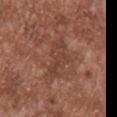Recorded during total-body skin imaging; not selected for excision or biopsy. Captured under white-light illumination. A male patient aged 43 to 47. Cropped from a whole-body photographic skin survey; the tile spans about 15 mm. The lesion-visualizer software estimated an average lesion color of about L≈42 a*≈22 b*≈26 (CIELAB), roughly 7 lightness units darker than nearby skin, and a lesion-to-skin contrast of about 5.5 (normalized; higher = more distinct). And it measured a border-irregularity index near 4.5/10. The lesion is located on the chest.